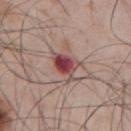biopsy status — no biopsy performed (imaged during a skin exam); patient — male, aged 53–57; illumination — white-light; site — the mid back; lesion size — ~4.5 mm (longest diameter); image source — ~15 mm crop, total-body skin-cancer survey; automated lesion analysis — a nevus-likeness score of about 0/100 and a detector confidence of about 100 out of 100 that the crop contains a lesion.